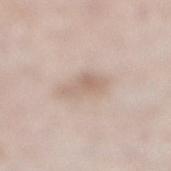This lesion was catalogued during total-body skin photography and was not selected for biopsy. Approximately 3 mm at its widest. A male patient, aged 58 to 62. The lesion-visualizer software estimated border irregularity of about 5 on a 0–10 scale and peripheral color asymmetry of about 0.5. The analysis additionally found a classifier nevus-likeness of about 5/100. A close-up tile cropped from a whole-body skin photograph, about 15 mm across. On the right lower leg. Imaged with white-light lighting.